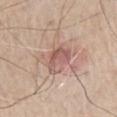{
  "biopsy_status": "not biopsied; imaged during a skin examination",
  "site": "chest",
  "automated_metrics": {
    "area_mm2_approx": 7.0,
    "eccentricity": 0.8,
    "shape_asymmetry": 0.35,
    "cielab_L": 57,
    "cielab_a": 21,
    "cielab_b": 24,
    "vs_skin_darker_L": 10.0,
    "vs_skin_contrast_norm": 6.5
  },
  "lighting": "white-light",
  "lesion_size": {
    "long_diameter_mm_approx": 4.0
  },
  "patient": {
    "sex": "male",
    "age_approx": 70
  },
  "image": {
    "source": "total-body photography crop",
    "field_of_view_mm": 15
  }
}